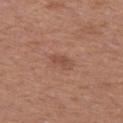notes: total-body-photography surveillance lesion; no biopsy
patient: female, aged 38 to 42
site: the left thigh
image-analysis metrics: a footprint of about 3.5 mm², an eccentricity of roughly 0.8, and a shape-asymmetry score of about 0.25 (0 = symmetric); a nevus-likeness score of about 5/100
size: ~2.5 mm (longest diameter)
acquisition: 15 mm crop, total-body photography